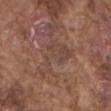The lesion was photographed on a routine skin check and not biopsied; there is no pathology result.
A region of skin cropped from a whole-body photographic capture, roughly 15 mm wide.
The lesion is located on the mid back.
Measured at roughly 6 mm in maximum diameter.
The tile uses white-light illumination.
A male patient, approximately 75 years of age.